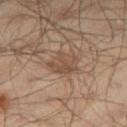The lesion was tiled from a total-body skin photograph and was not biopsied.
Captured under cross-polarized illumination.
On the right thigh.
A male patient, approximately 65 years of age.
A 15 mm close-up extracted from a 3D total-body photography capture.